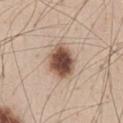Clinical impression:
Part of a total-body skin-imaging series; this lesion was reviewed on a skin check and was not flagged for biopsy.
Image and clinical context:
A 15 mm crop from a total-body photograph taken for skin-cancer surveillance. A male subject, about 45 years old. The tile uses white-light illumination. The lesion is on the front of the torso.